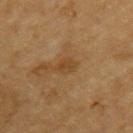Assessment:
The lesion was tiled from a total-body skin photograph and was not biopsied.
Image and clinical context:
The subject is a male aged approximately 85 or older. Cropped from a total-body skin-imaging series; the visible field is about 15 mm. Longest diameter approximately 2.5 mm. The total-body-photography lesion software estimated a nevus-likeness score of about 0/100 and a lesion-detection confidence of about 100/100. From the upper back.